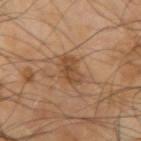image-analysis metrics = a border-irregularity rating of about 3/10 and a color-variation rating of about 3.5/10; a classifier nevus-likeness of about 30/100 and lesion-presence confidence of about 100/100
patient = male, aged approximately 65
body site = the right upper arm
illumination = cross-polarized illumination
image = 15 mm crop, total-body photography
lesion diameter = about 3.5 mm